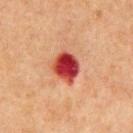Assessment:
This lesion was catalogued during total-body skin photography and was not selected for biopsy.
Context:
An algorithmic analysis of the crop reported a mean CIELAB color near L≈39 a*≈37 b*≈29, about 19 CIELAB-L* units darker than the surrounding skin, and a normalized lesion–skin contrast near 14.5. And it measured a classifier nevus-likeness of about 0/100. Approximately 4 mm at its widest. A male patient, aged around 65. Cropped from a whole-body photographic skin survey; the tile spans about 15 mm. The lesion is on the chest.